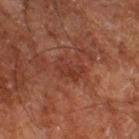workup: catalogued during a skin exam; not biopsied | lighting: cross-polarized illumination | subject: aged around 65 | acquisition: ~15 mm tile from a whole-body skin photo | location: the leg | lesion size: ~3.5 mm (longest diameter).